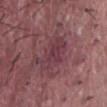Assessment:
No biopsy was performed on this lesion — it was imaged during a full skin examination and was not determined to be concerning.